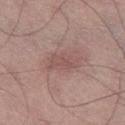Case summary:
- patient: male, roughly 55 years of age
- tile lighting: white-light
- automated metrics: an area of roughly 6.5 mm², an eccentricity of roughly 0.85, and a symmetry-axis asymmetry near 0.3; an automated nevus-likeness rating near 0 out of 100 and a lesion-detection confidence of about 100/100
- image: ~15 mm crop, total-body skin-cancer survey
- location: the leg